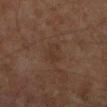<tbp_lesion>
<biopsy_status>not biopsied; imaged during a skin examination</biopsy_status>
<patient>
  <sex>male</sex>
  <age_approx>60</age_approx>
</patient>
<automated_metrics>
  <eccentricity>0.85</eccentricity>
  <shape_asymmetry>0.4</shape_asymmetry>
  <border_irregularity_0_10>4.0</border_irregularity_0_10>
  <color_variation_0_10>1.5</color_variation_0_10>
  <peripheral_color_asymmetry>0.5</peripheral_color_asymmetry>
  <nevus_likeness_0_100>0</nevus_likeness_0_100>
</automated_metrics>
<image>
  <source>total-body photography crop</source>
  <field_of_view_mm>15</field_of_view_mm>
</image>
<lesion_size>
  <long_diameter_mm_approx>3.0</long_diameter_mm_approx>
</lesion_size>
<site>left lower leg</site>
</tbp_lesion>